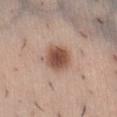follow-up: imaged on a skin check; not biopsied
anatomic site: the abdomen
patient: female, about 30 years old
image source: total-body-photography crop, ~15 mm field of view